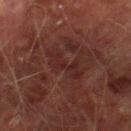Assessment:
Imaged during a routine full-body skin examination; the lesion was not biopsied and no histopathology is available.
Image and clinical context:
Imaged with cross-polarized lighting. Measured at roughly 5.5 mm in maximum diameter. A 15 mm close-up tile from a total-body photography series done for melanoma screening. The subject is a male aged approximately 75. The lesion is on the right thigh.